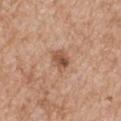workup=imaged on a skin check; not biopsied | lighting=white-light | anatomic site=the right upper arm | automated metrics=a border-irregularity index near 2.5/10 and a color-variation rating of about 6/10 | image source=15 mm crop, total-body photography | diameter=about 3 mm | patient=male, in their mid-60s.